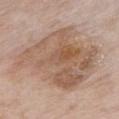The lesion was photographed on a routine skin check and not biopsied; there is no pathology result.
Captured under white-light illumination.
A roughly 15 mm field-of-view crop from a total-body skin photograph.
A female patient, in their mid-80s or older.
The total-body-photography lesion software estimated a lesion area of about 55 mm², a shape eccentricity near 0.65, and a symmetry-axis asymmetry near 0.3. It also reported a mean CIELAB color near L≈57 a*≈17 b*≈29, a lesion–skin lightness drop of about 10, and a lesion-to-skin contrast of about 7 (normalized; higher = more distinct).
From the chest.